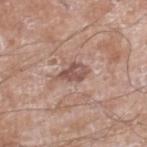Q: What is the imaging modality?
A: total-body-photography crop, ~15 mm field of view
Q: What are the patient's age and sex?
A: male, about 60 years old
Q: Where on the body is the lesion?
A: the leg